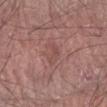| key | value |
|---|---|
| notes | no biopsy performed (imaged during a skin exam) |
| lighting | white-light illumination |
| patient | male, aged 63–67 |
| anatomic site | the arm |
| lesion size | ~3.5 mm (longest diameter) |
| image-analysis metrics | a lesion area of about 4 mm², a shape eccentricity near 0.9, and a symmetry-axis asymmetry near 0.5; a color-variation rating of about 1/10; an automated nevus-likeness rating near 0 out of 100 and a detector confidence of about 90 out of 100 that the crop contains a lesion |
| image | total-body-photography crop, ~15 mm field of view |